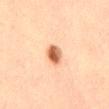Q: Was this lesion biopsied?
A: total-body-photography surveillance lesion; no biopsy
Q: What kind of image is this?
A: total-body-photography crop, ~15 mm field of view
Q: Who is the patient?
A: male, aged 58–62
Q: What is the anatomic site?
A: the mid back